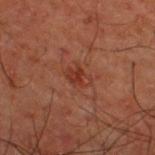Captured during whole-body skin photography for melanoma surveillance; the lesion was not biopsied. A 15 mm close-up tile from a total-body photography series done for melanoma screening. The subject is a male aged around 50. Located on the upper back.